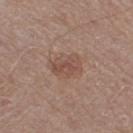biopsy status: no biopsy performed (imaged during a skin exam)
automated lesion analysis: a shape-asymmetry score of about 0.2 (0 = symmetric); a nevus-likeness score of about 5/100 and a lesion-detection confidence of about 100/100
patient: female, approximately 55 years of age
body site: the leg
tile lighting: white-light illumination
size: about 4 mm
imaging modality: ~15 mm tile from a whole-body skin photo